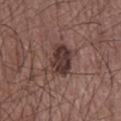{"biopsy_status": "not biopsied; imaged during a skin examination", "lesion_size": {"long_diameter_mm_approx": 4.0}, "patient": {"sex": "male", "age_approx": 60}, "image": {"source": "total-body photography crop", "field_of_view_mm": 15}, "site": "chest"}